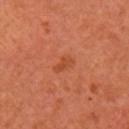Findings:
• follow-up: total-body-photography surveillance lesion; no biopsy
• patient: female, aged 63 to 67
• image source: 15 mm crop, total-body photography
• site: the right upper arm